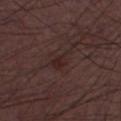Q: Is there a histopathology result?
A: no biopsy performed (imaged during a skin exam)
Q: Who is the patient?
A: male, approximately 50 years of age
Q: Lesion location?
A: the left thigh
Q: What is the imaging modality?
A: total-body-photography crop, ~15 mm field of view